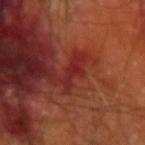Impression:
This lesion was catalogued during total-body skin photography and was not selected for biopsy.
Context:
A male subject about 60 years old. About 3 mm across. The lesion is on the arm. A 15 mm close-up extracted from a 3D total-body photography capture. Captured under cross-polarized illumination.